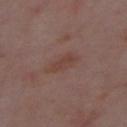Clinical impression:
The lesion was photographed on a routine skin check and not biopsied; there is no pathology result.
Clinical summary:
A 15 mm crop from a total-body photograph taken for skin-cancer surveillance. An algorithmic analysis of the crop reported a nevus-likeness score of about 0/100. Measured at roughly 3.5 mm in maximum diameter. A male subject aged approximately 50. On the chest.